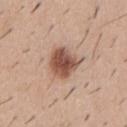{
  "biopsy_status": "not biopsied; imaged during a skin examination",
  "lighting": "white-light",
  "image": {
    "source": "total-body photography crop",
    "field_of_view_mm": 15
  },
  "site": "chest",
  "automated_metrics": {
    "border_irregularity_0_10": 1.5,
    "peripheral_color_asymmetry": 1.5
  },
  "lesion_size": {
    "long_diameter_mm_approx": 3.5
  },
  "patient": {
    "sex": "male",
    "age_approx": 40
  }
}